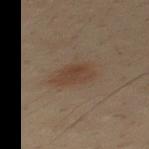The lesion was photographed on a routine skin check and not biopsied; there is no pathology result. Automated image analysis of the tile measured a lesion area of about 7 mm². It also reported a mean CIELAB color near L≈32 a*≈13 b*≈22 and a lesion–skin lightness drop of about 6. And it measured border irregularity of about 2.5 on a 0–10 scale, a within-lesion color-variation index near 2/10, and radial color variation of about 0.5. About 3.5 mm across. The tile uses cross-polarized illumination. A male subject, in their 30s. Located on the mid back. A close-up tile cropped from a whole-body skin photograph, about 15 mm across.